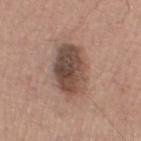Q: Was a biopsy performed?
A: imaged on a skin check; not biopsied
Q: How was the tile lit?
A: white-light illumination
Q: Where on the body is the lesion?
A: the left thigh
Q: How was this image acquired?
A: 15 mm crop, total-body photography
Q: Automated lesion metrics?
A: a shape eccentricity near 0.8 and a shape-asymmetry score of about 0.1 (0 = symmetric); roughly 14 lightness units darker than nearby skin; lesion-presence confidence of about 100/100
Q: Who is the patient?
A: male, about 75 years old
Q: What is the lesion's diameter?
A: ≈6 mm